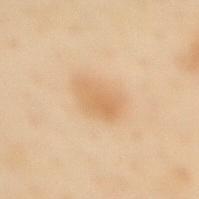Q: Was a biopsy performed?
A: imaged on a skin check; not biopsied
Q: What did automated image analysis measure?
A: a border-irregularity rating of about 2/10 and peripheral color asymmetry of about 0.5; an automated nevus-likeness rating near 45 out of 100 and lesion-presence confidence of about 100/100
Q: How was the tile lit?
A: cross-polarized
Q: What are the patient's age and sex?
A: female, aged approximately 60
Q: What is the lesion's diameter?
A: about 4.5 mm
Q: How was this image acquired?
A: ~15 mm crop, total-body skin-cancer survey
Q: Lesion location?
A: the upper back